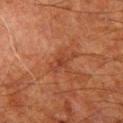Recorded during total-body skin imaging; not selected for excision or biopsy. This image is a 15 mm lesion crop taken from a total-body photograph. Located on the left thigh. An algorithmic analysis of the crop reported a color-variation rating of about 3.5/10 and a peripheral color-asymmetry measure near 1. The analysis additionally found an automated nevus-likeness rating near 0 out of 100 and lesion-presence confidence of about 100/100. A male subject about 80 years old. This is a cross-polarized tile.